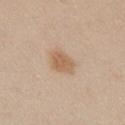site: chest
lesion_size:
  long_diameter_mm_approx: 4.0
image:
  source: total-body photography crop
  field_of_view_mm: 15
lighting: white-light
patient:
  sex: female
  age_approx: 40
automated_metrics:
  border_irregularity_0_10: 2.5
  color_variation_0_10: 2.5
  peripheral_color_asymmetry: 1.0